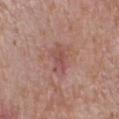<case>
<biopsy_status>not biopsied; imaged during a skin examination</biopsy_status>
<automated_metrics>
  <area_mm2_approx>5.0</area_mm2_approx>
  <cielab_L>50</cielab_L>
  <cielab_a>24</cielab_a>
  <cielab_b>23</cielab_b>
  <vs_skin_darker_L>8.0</vs_skin_darker_L>
  <vs_skin_contrast_norm>5.5</vs_skin_contrast_norm>
  <nevus_likeness_0_100>0</nevus_likeness_0_100>
  <lesion_detection_confidence_0_100>100</lesion_detection_confidence_0_100>
</automated_metrics>
<patient>
  <sex>male</sex>
  <age_approx>55</age_approx>
</patient>
<image>
  <source>total-body photography crop</source>
  <field_of_view_mm>15</field_of_view_mm>
</image>
<lesion_size>
  <long_diameter_mm_approx>3.0</long_diameter_mm_approx>
</lesion_size>
<lighting>white-light</lighting>
<site>right forearm</site>
</case>